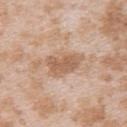Findings:
• follow-up — imaged on a skin check; not biopsied
• location — the upper back
• subject — female, approximately 25 years of age
• image source — ~15 mm tile from a whole-body skin photo
• tile lighting — white-light
• size — about 4.5 mm